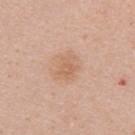workup: catalogued during a skin exam; not biopsied | body site: the right upper arm | patient: female, aged approximately 30 | imaging modality: ~15 mm crop, total-body skin-cancer survey.